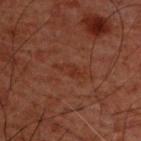biopsy status = imaged on a skin check; not biopsied
acquisition = 15 mm crop, total-body photography
illumination = cross-polarized
lesion size = about 3 mm
body site = the upper back
subject = male, about 60 years old